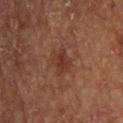workup: catalogued during a skin exam; not biopsied | subject: male, aged 53 to 57 | location: the upper back | image source: 15 mm crop, total-body photography | image-analysis metrics: an area of roughly 4.5 mm² and a shape-asymmetry score of about 0.25 (0 = symmetric); a lesion color around L≈30 a*≈20 b*≈25 in CIELAB; an automated nevus-likeness rating near 0 out of 100 and a lesion-detection confidence of about 100/100 | size: ≈2.5 mm.